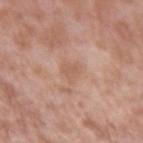{"site": "arm", "image": {"source": "total-body photography crop", "field_of_view_mm": 15}, "patient": {"sex": "male", "age_approx": 55}}